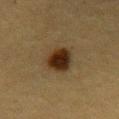follow-up: total-body-photography surveillance lesion; no biopsy
image: total-body-photography crop, ~15 mm field of view
anatomic site: the chest
subject: female, aged 53–57